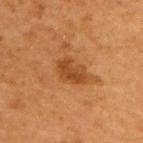notes: imaged on a skin check; not biopsied
body site: the arm
lesion size: about 4 mm
tile lighting: cross-polarized illumination
image: ~15 mm crop, total-body skin-cancer survey
automated lesion analysis: an area of roughly 6.5 mm², a shape eccentricity near 0.8, and a symmetry-axis asymmetry near 0.3; a border-irregularity index near 3/10, a within-lesion color-variation index near 2.5/10, and peripheral color asymmetry of about 0.5
patient: male, aged 63 to 67